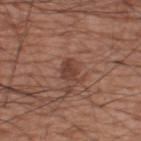<tbp_lesion>
  <biopsy_status>not biopsied; imaged during a skin examination</biopsy_status>
  <image>
    <source>total-body photography crop</source>
    <field_of_view_mm>15</field_of_view_mm>
  </image>
  <lighting>white-light</lighting>
  <site>upper back</site>
  <lesion_size>
    <long_diameter_mm_approx>3.0</long_diameter_mm_approx>
  </lesion_size>
  <patient>
    <sex>male</sex>
    <age_approx>60</age_approx>
  </patient>
</tbp_lesion>